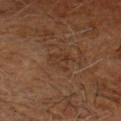biopsy status — total-body-photography surveillance lesion; no biopsy
diameter — ≈3 mm
patient — male, aged approximately 65
image — 15 mm crop, total-body photography
tile lighting — cross-polarized illumination